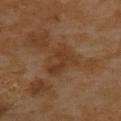<case>
<biopsy_status>not biopsied; imaged during a skin examination</biopsy_status>
<patient>
  <sex>female</sex>
  <age_approx>55</age_approx>
</patient>
<image>
  <source>total-body photography crop</source>
  <field_of_view_mm>15</field_of_view_mm>
</image>
<site>upper back</site>
<automated_metrics>
  <cielab_L>38</cielab_L>
  <cielab_a>19</cielab_a>
  <cielab_b>32</cielab_b>
  <vs_skin_darker_L>7.0</vs_skin_darker_L>
  <vs_skin_contrast_norm>6.0</vs_skin_contrast_norm>
  <border_irregularity_0_10>3.5</border_irregularity_0_10>
  <peripheral_color_asymmetry>1.0</peripheral_color_asymmetry>
</automated_metrics>
<lesion_size>
  <long_diameter_mm_approx>4.0</long_diameter_mm_approx>
</lesion_size>
<lighting>cross-polarized</lighting>
</case>